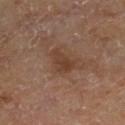notes: no biopsy performed (imaged during a skin exam); image source: ~15 mm tile from a whole-body skin photo; size: about 3.5 mm; tile lighting: cross-polarized; subject: male, aged around 65; body site: the leg.